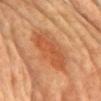Clinical impression:
Recorded during total-body skin imaging; not selected for excision or biopsy.
Context:
Cropped from a whole-body photographic skin survey; the tile spans about 15 mm. About 7.5 mm across. An algorithmic analysis of the crop reported a footprint of about 26 mm², an eccentricity of roughly 0.75, and a shape-asymmetry score of about 0.2 (0 = symmetric). It also reported a mean CIELAB color near L≈55 a*≈26 b*≈39, a lesion–skin lightness drop of about 8, and a lesion-to-skin contrast of about 6 (normalized; higher = more distinct). The software also gave a border-irregularity index near 3/10 and internal color variation of about 5.5 on a 0–10 scale. The analysis additionally found a classifier nevus-likeness of about 90/100 and a lesion-detection confidence of about 100/100. A female subject, aged approximately 65. The lesion is on the chest. Imaged with cross-polarized lighting.